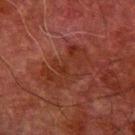This lesion was catalogued during total-body skin photography and was not selected for biopsy.
A male patient in their 80s.
Imaged with cross-polarized lighting.
A lesion tile, about 15 mm wide, cut from a 3D total-body photograph.
An algorithmic analysis of the crop reported a lesion area of about 16 mm², a shape eccentricity near 0.85, and a shape-asymmetry score of about 0.35 (0 = symmetric).
The lesion is located on the arm.
The recorded lesion diameter is about 6.5 mm.